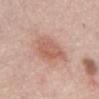follow-up: no biopsy performed (imaged during a skin exam) | tile lighting: white-light | image-analysis metrics: an area of roughly 12 mm² and an outline eccentricity of about 0.65 (0 = round, 1 = elongated); an average lesion color of about L≈59 a*≈23 b*≈28 (CIELAB) and a normalized border contrast of about 6; a within-lesion color-variation index near 3/10 and peripheral color asymmetry of about 1; an automated nevus-likeness rating near 70 out of 100 and lesion-presence confidence of about 100/100 | lesion diameter: ~4.5 mm (longest diameter) | body site: the abdomen | acquisition: 15 mm crop, total-body photography | patient: female, aged around 65.